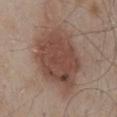Findings:
– workup: catalogued during a skin exam; not biopsied
– location: the mid back
– patient: male, in their mid-50s
– imaging modality: 15 mm crop, total-body photography